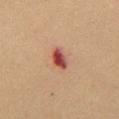Captured during whole-body skin photography for melanoma surveillance; the lesion was not biopsied.